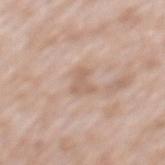image: total-body-photography crop, ~15 mm field of view | patient: male, aged 48–52 | site: the mid back | size: about 3 mm | illumination: white-light | image-analysis metrics: internal color variation of about 1.5 on a 0–10 scale and a peripheral color-asymmetry measure near 0.5; an automated nevus-likeness rating near 0 out of 100 and a detector confidence of about 100 out of 100 that the crop contains a lesion.